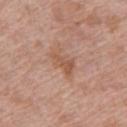Recorded during total-body skin imaging; not selected for excision or biopsy.
From the left upper arm.
A female patient aged around 60.
A roughly 15 mm field-of-view crop from a total-body skin photograph.